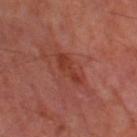notes: imaged on a skin check; not biopsied
illumination: cross-polarized illumination
patient: male, approximately 70 years of age
site: the left thigh
acquisition: total-body-photography crop, ~15 mm field of view